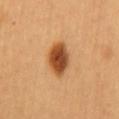Part of a total-body skin-imaging series; this lesion was reviewed on a skin check and was not flagged for biopsy. The patient is a female in their 60s. This is a cross-polarized tile. A 15 mm close-up tile from a total-body photography series done for melanoma screening. Measured at roughly 4.5 mm in maximum diameter. On the back.